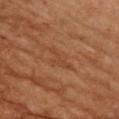Image and clinical context:
Automated tile analysis of the lesion measured a classifier nevus-likeness of about 0/100 and lesion-presence confidence of about 85/100. A roughly 15 mm field-of-view crop from a total-body skin photograph. A male subject aged approximately 70. On the chest.